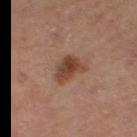| field | value |
|---|---|
| biopsy status | total-body-photography surveillance lesion; no biopsy |
| patient | male, aged 53–57 |
| lesion size | about 4 mm |
| location | the right thigh |
| imaging modality | 15 mm crop, total-body photography |